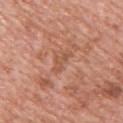Assessment: The lesion was photographed on a routine skin check and not biopsied; there is no pathology result. Image and clinical context: Imaged with white-light lighting. Automated tile analysis of the lesion measured a classifier nevus-likeness of about 0/100 and a lesion-detection confidence of about 90/100. A male patient, aged 58 to 62. Located on the upper back. A 15 mm crop from a total-body photograph taken for skin-cancer surveillance.